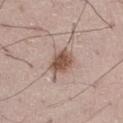Assessment: Part of a total-body skin-imaging series; this lesion was reviewed on a skin check and was not flagged for biopsy. Clinical summary: On the right thigh. The lesion-visualizer software estimated a mean CIELAB color near L≈52 a*≈18 b*≈25, about 14 CIELAB-L* units darker than the surrounding skin, and a normalized border contrast of about 9.5. And it measured border irregularity of about 2 on a 0–10 scale and peripheral color asymmetry of about 1. It also reported an automated nevus-likeness rating near 90 out of 100 and lesion-presence confidence of about 100/100. The patient is a male approximately 50 years of age. This is a white-light tile. A 15 mm close-up extracted from a 3D total-body photography capture.